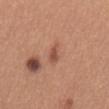Assessment: Recorded during total-body skin imaging; not selected for excision or biopsy. Context: The subject is a female aged around 40. Captured under white-light illumination. The lesion's longest dimension is about 3 mm. A 15 mm close-up tile from a total-body photography series done for melanoma screening. Automated tile analysis of the lesion measured an area of roughly 3 mm², a shape eccentricity near 0.85, and a symmetry-axis asymmetry near 0.4. It also reported a lesion color around L≈52 a*≈25 b*≈30 in CIELAB, a lesion–skin lightness drop of about 10, and a lesion-to-skin contrast of about 6.5 (normalized; higher = more distinct). It also reported a border-irregularity rating of about 4/10 and a color-variation rating of about 1/10. The lesion is located on the front of the torso.